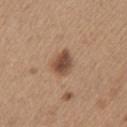Background:
The total-body-photography lesion software estimated a lesion area of about 6 mm², a shape eccentricity near 0.65, and a symmetry-axis asymmetry near 0.25. It also reported a border-irregularity index near 2/10, a within-lesion color-variation index near 4/10, and a peripheral color-asymmetry measure near 1.5. And it measured a classifier nevus-likeness of about 95/100. The lesion's longest dimension is about 3 mm. A male patient, aged approximately 65. The lesion is located on the left upper arm. This is a white-light tile. Cropped from a total-body skin-imaging series; the visible field is about 15 mm.